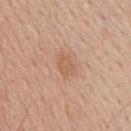Q: Was a biopsy performed?
A: imaged on a skin check; not biopsied
Q: How large is the lesion?
A: ≈2.5 mm
Q: Patient demographics?
A: male, roughly 60 years of age
Q: How was this image acquired?
A: total-body-photography crop, ~15 mm field of view
Q: Lesion location?
A: the back
Q: Illumination type?
A: white-light illumination